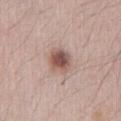This lesion was catalogued during total-body skin photography and was not selected for biopsy. A female patient, about 20 years old. On the abdomen. A region of skin cropped from a whole-body photographic capture, roughly 15 mm wide.